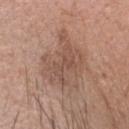Q: Was this lesion biopsied?
A: imaged on a skin check; not biopsied
Q: What kind of image is this?
A: ~15 mm crop, total-body skin-cancer survey
Q: How was the tile lit?
A: white-light illumination
Q: Patient demographics?
A: male, aged approximately 55
Q: What is the lesion's diameter?
A: ~7 mm (longest diameter)